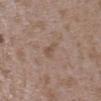Q: What lighting was used for the tile?
A: white-light
Q: How large is the lesion?
A: about 2.5 mm
Q: What did automated image analysis measure?
A: an area of roughly 2.5 mm², an outline eccentricity of about 0.85 (0 = round, 1 = elongated), and a shape-asymmetry score of about 0.45 (0 = symmetric); a mean CIELAB color near L≈51 a*≈16 b*≈26, roughly 6 lightness units darker than nearby skin, and a normalized lesion–skin contrast near 5; a border-irregularity index near 4/10; a classifier nevus-likeness of about 0/100 and a lesion-detection confidence of about 100/100
Q: What is the anatomic site?
A: the upper back
Q: How was this image acquired?
A: ~15 mm tile from a whole-body skin photo
Q: Patient demographics?
A: female, in their mid- to late 30s